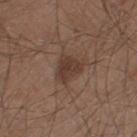The lesion was photographed on a routine skin check and not biopsied; there is no pathology result.
The lesion is on the left thigh.
Approximately 3.5 mm at its widest.
A male patient in their mid-40s.
The lesion-visualizer software estimated a border-irregularity rating of about 3.5/10, a within-lesion color-variation index near 2.5/10, and peripheral color asymmetry of about 0.5. The analysis additionally found an automated nevus-likeness rating near 75 out of 100 and a detector confidence of about 100 out of 100 that the crop contains a lesion.
A 15 mm close-up tile from a total-body photography series done for melanoma screening.
This is a white-light tile.